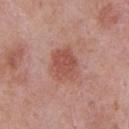From the front of the torso.
Cropped from a whole-body photographic skin survey; the tile spans about 15 mm.
About 4 mm across.
A male patient, in their mid-50s.